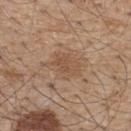biopsy status: no biopsy performed (imaged during a skin exam); illumination: white-light; acquisition: total-body-photography crop, ~15 mm field of view; site: the upper back; subject: male, in their mid- to late 50s; lesion diameter: ≈4 mm.